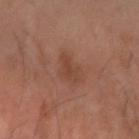Impression: Captured during whole-body skin photography for melanoma surveillance; the lesion was not biopsied. Background: The lesion is on the right forearm. A male subject, aged approximately 60. Cropped from a total-body skin-imaging series; the visible field is about 15 mm. Longest diameter approximately 3 mm.